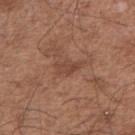Q: Is there a histopathology result?
A: catalogued during a skin exam; not biopsied
Q: Lesion location?
A: the right upper arm
Q: Lesion size?
A: about 3.5 mm
Q: How was the tile lit?
A: white-light illumination
Q: Who is the patient?
A: male, aged around 50
Q: What is the imaging modality?
A: 15 mm crop, total-body photography